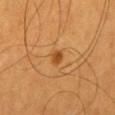Findings:
* workup · catalogued during a skin exam; not biopsied
* subject · male, aged 58–62
* tile lighting · cross-polarized illumination
* size · about 2 mm
* body site · the mid back
* acquisition · total-body-photography crop, ~15 mm field of view